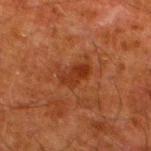follow-up = catalogued during a skin exam; not biopsied | lighting = cross-polarized | location = the left lower leg | automated metrics = a mean CIELAB color near L≈27 a*≈24 b*≈30 and a normalized lesion–skin contrast near 7; an automated nevus-likeness rating near 35 out of 100 and lesion-presence confidence of about 100/100 | imaging modality = ~15 mm crop, total-body skin-cancer survey | diameter = ~3.5 mm (longest diameter) | subject = male, aged approximately 80.